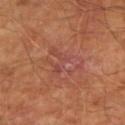follow-up: no biopsy performed (imaged during a skin exam) | patient: male, aged 63–67 | lighting: cross-polarized illumination | automated metrics: a lesion color around L≈46 a*≈25 b*≈27 in CIELAB, a lesion–skin lightness drop of about 4, and a normalized lesion–skin contrast near 4.5; border irregularity of about 5.5 on a 0–10 scale and a color-variation rating of about 6/10; a nevus-likeness score of about 0/100 and lesion-presence confidence of about 95/100 | image source: 15 mm crop, total-body photography | site: the right upper arm | lesion diameter: ≈4.5 mm.